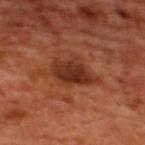This lesion was catalogued during total-body skin photography and was not selected for biopsy.
A male subject, approximately 50 years of age.
The lesion is on the upper back.
An algorithmic analysis of the crop reported border irregularity of about 3 on a 0–10 scale, a color-variation rating of about 4/10, and radial color variation of about 1.5.
The recorded lesion diameter is about 5 mm.
A region of skin cropped from a whole-body photographic capture, roughly 15 mm wide.